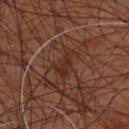Imaged during a routine full-body skin examination; the lesion was not biopsied and no histopathology is available.
An algorithmic analysis of the crop reported roughly 6 lightness units darker than nearby skin and a normalized lesion–skin contrast near 6.5. And it measured a border-irregularity rating of about 5/10, a within-lesion color-variation index near 2/10, and peripheral color asymmetry of about 0.5. The software also gave a nevus-likeness score of about 0/100.
A male subject roughly 45 years of age.
A region of skin cropped from a whole-body photographic capture, roughly 15 mm wide.
The lesion is located on the upper back.
The recorded lesion diameter is about 3.5 mm.